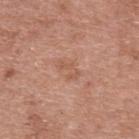This lesion was catalogued during total-body skin photography and was not selected for biopsy. A female subject, about 70 years old. A 15 mm close-up extracted from a 3D total-body photography capture. The lesion is on the upper back.